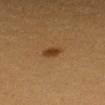No biopsy was performed on this lesion — it was imaged during a full skin examination and was not determined to be concerning.
A 15 mm close-up extracted from a 3D total-body photography capture.
On the left forearm.
The recorded lesion diameter is about 2.5 mm.
An algorithmic analysis of the crop reported an area of roughly 3.5 mm² and an outline eccentricity of about 0.75 (0 = round, 1 = elongated). The analysis additionally found a lesion color around L≈36 a*≈19 b*≈33 in CIELAB, a lesion–skin lightness drop of about 10, and a normalized border contrast of about 9. The analysis additionally found a border-irregularity index near 1.5/10, a within-lesion color-variation index near 3/10, and peripheral color asymmetry of about 1.
The subject is a female aged 28–32.
This is a cross-polarized tile.